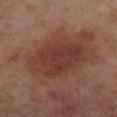Clinical impression:
Captured during whole-body skin photography for melanoma surveillance; the lesion was not biopsied.
Image and clinical context:
From the left lower leg. Imaged with cross-polarized lighting. Longest diameter approximately 6.5 mm. A female patient approximately 55 years of age. Cropped from a whole-body photographic skin survey; the tile spans about 15 mm.